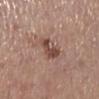Case summary:
– tile lighting — white-light
– patient — female, in their mid-60s
– size — about 3 mm
– image — ~15 mm tile from a whole-body skin photo
– body site — the left lower leg